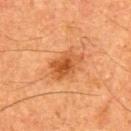| key | value |
|---|---|
| notes | imaged on a skin check; not biopsied |
| subject | male, aged 63 to 67 |
| imaging modality | ~15 mm tile from a whole-body skin photo |
| body site | the back |